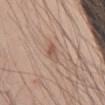Q: Is there a histopathology result?
A: no biopsy performed (imaged during a skin exam)